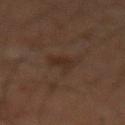  automated_metrics:
    area_mm2_approx: 3.5
    eccentricity: 0.65
    shape_asymmetry: 0.4
  site: mid back
  lesion_size:
    long_diameter_mm_approx: 2.5
  lighting: cross-polarized
  patient:
    sex: male
    age_approx: 60
  image:
    source: total-body photography crop
    field_of_view_mm: 15